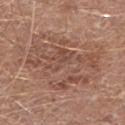workup: no biopsy performed (imaged during a skin exam)
patient: male, aged 78–82
illumination: white-light
TBP lesion metrics: a footprint of about 42 mm² and an outline eccentricity of about 0.8 (0 = round, 1 = elongated)
site: the right lower leg
diameter: about 9.5 mm
imaging modality: ~15 mm tile from a whole-body skin photo